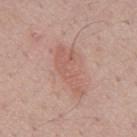biopsy_status: not biopsied; imaged during a skin examination
lesion_size:
  long_diameter_mm_approx: 6.5
site: mid back
patient:
  sex: male
  age_approx: 45
image:
  source: total-body photography crop
  field_of_view_mm: 15
lighting: white-light